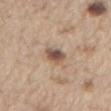- anatomic site — the abdomen
- tile lighting — white-light illumination
- image — ~15 mm crop, total-body skin-cancer survey
- patient — male, about 70 years old
- size — ~3 mm (longest diameter)